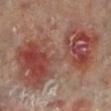Q: Was a biopsy performed?
A: no biopsy performed (imaged during a skin exam)
Q: How was this image acquired?
A: total-body-photography crop, ~15 mm field of view
Q: Where on the body is the lesion?
A: the left lower leg
Q: Patient demographics?
A: female, about 60 years old
Q: How was the tile lit?
A: cross-polarized
Q: What did automated image analysis measure?
A: an area of roughly 55 mm² and two-axis asymmetry of about 0.3; a mean CIELAB color near L≈45 a*≈24 b*≈25, a lesion–skin lightness drop of about 10, and a normalized lesion–skin contrast near 8; a border-irregularity rating of about 7/10, a color-variation rating of about 8/10, and radial color variation of about 2.5; an automated nevus-likeness rating near 0 out of 100
Q: What is the lesion's diameter?
A: ≈13 mm